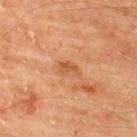Recorded during total-body skin imaging; not selected for excision or biopsy. Cropped from a total-body skin-imaging series; the visible field is about 15 mm. Measured at roughly 2.5 mm in maximum diameter. Automated image analysis of the tile measured a mean CIELAB color near L≈55 a*≈26 b*≈41 and a lesion-to-skin contrast of about 6.5 (normalized; higher = more distinct). It also reported border irregularity of about 4 on a 0–10 scale and internal color variation of about 2.5 on a 0–10 scale. And it measured an automated nevus-likeness rating near 0 out of 100. The subject is a male roughly 65 years of age. Imaged with cross-polarized lighting. Located on the back.